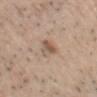Impression:
This lesion was catalogued during total-body skin photography and was not selected for biopsy.
Background:
Approximately 3 mm at its widest. The patient is a male aged 33 to 37. Located on the head or neck. This is a white-light tile. Cropped from a total-body skin-imaging series; the visible field is about 15 mm.